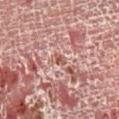The lesion was tiled from a total-body skin photograph and was not biopsied.
A 15 mm crop from a total-body photograph taken for skin-cancer surveillance.
The lesion is located on the right lower leg.
A male subject aged around 55.